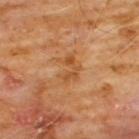• notes · imaged on a skin check; not biopsied
• image source · ~15 mm crop, total-body skin-cancer survey
• anatomic site · the chest
• patient · male, aged 58–62